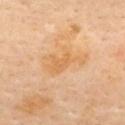Assessment: The lesion was tiled from a total-body skin photograph and was not biopsied. Clinical summary: The tile uses cross-polarized illumination. The lesion-visualizer software estimated an average lesion color of about L≈68 a*≈22 b*≈44 (CIELAB), about 7 CIELAB-L* units darker than the surrounding skin, and a lesion-to-skin contrast of about 6 (normalized; higher = more distinct). It also reported border irregularity of about 6 on a 0–10 scale, a color-variation rating of about 1.5/10, and radial color variation of about 0.5. Cropped from a total-body skin-imaging series; the visible field is about 15 mm. Measured at roughly 5 mm in maximum diameter. A female subject in their 60s. Located on the back.